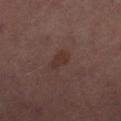Q: Was a biopsy performed?
A: catalogued during a skin exam; not biopsied
Q: What is the lesion's diameter?
A: about 3 mm
Q: Lesion location?
A: the leg
Q: Automated lesion metrics?
A: an eccentricity of roughly 0.75 and two-axis asymmetry of about 0.3; a border-irregularity rating of about 3/10, internal color variation of about 1.5 on a 0–10 scale, and peripheral color asymmetry of about 0.5
Q: What kind of image is this?
A: ~15 mm tile from a whole-body skin photo
Q: Who is the patient?
A: female, about 50 years old
Q: How was the tile lit?
A: cross-polarized illumination